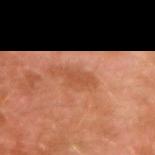The lesion was tiled from a total-body skin photograph and was not biopsied. The tile uses cross-polarized illumination. The lesion is on the left arm. A lesion tile, about 15 mm wide, cut from a 3D total-body photograph. A male patient, about 30 years old.